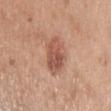Impression:
Recorded during total-body skin imaging; not selected for excision or biopsy.
Clinical summary:
On the upper back. Imaged with white-light lighting. The lesion-visualizer software estimated a footprint of about 11 mm², a shape eccentricity near 0.85, and a shape-asymmetry score of about 0.2 (0 = symmetric). The analysis additionally found a lesion color around L≈55 a*≈23 b*≈29 in CIELAB, about 11 CIELAB-L* units darker than the surrounding skin, and a normalized lesion–skin contrast near 7.5. Cropped from a total-body skin-imaging series; the visible field is about 15 mm. A male patient approximately 40 years of age.